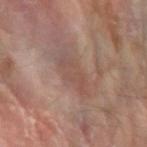* follow-up · catalogued during a skin exam; not biopsied
* subject · male, aged around 85 or older
* site · the left forearm
* imaging modality · total-body-photography crop, ~15 mm field of view